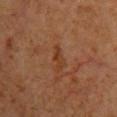The lesion was photographed on a routine skin check and not biopsied; there is no pathology result. About 3 mm across. Captured under cross-polarized illumination. Located on the chest. The patient is a female aged approximately 60. A lesion tile, about 15 mm wide, cut from a 3D total-body photograph.